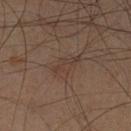notes: imaged on a skin check; not biopsied
location: the right lower leg
imaging modality: total-body-photography crop, ~15 mm field of view
patient: male, aged 58 to 62